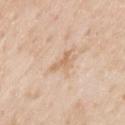Q: Is there a histopathology result?
A: imaged on a skin check; not biopsied
Q: Patient demographics?
A: male, in their 80s
Q: Illumination type?
A: white-light
Q: How was this image acquired?
A: 15 mm crop, total-body photography
Q: Lesion location?
A: the back
Q: How large is the lesion?
A: about 3 mm